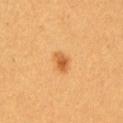Q: Is there a histopathology result?
A: catalogued during a skin exam; not biopsied
Q: Patient demographics?
A: female, roughly 30 years of age
Q: How was this image acquired?
A: ~15 mm tile from a whole-body skin photo
Q: Where on the body is the lesion?
A: the left upper arm
Q: How large is the lesion?
A: about 2.5 mm
Q: What did automated image analysis measure?
A: a lesion area of about 3.5 mm², an eccentricity of roughly 0.75, and two-axis asymmetry of about 0.25; a border-irregularity rating of about 2/10 and a peripheral color-asymmetry measure near 1.5; a nevus-likeness score of about 95/100